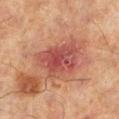Q: Was a biopsy performed?
A: total-body-photography surveillance lesion; no biopsy
Q: What kind of image is this?
A: total-body-photography crop, ~15 mm field of view
Q: What is the anatomic site?
A: the right lower leg
Q: What is the lesion's diameter?
A: ~5.5 mm (longest diameter)
Q: What lighting was used for the tile?
A: cross-polarized illumination
Q: Patient demographics?
A: male, in their mid- to late 60s